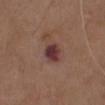The lesion was photographed on a routine skin check and not biopsied; there is no pathology result. This image is a 15 mm lesion crop taken from a total-body photograph. The tile uses white-light illumination. About 3 mm across. A male subject, about 75 years old. The total-body-photography lesion software estimated a lesion area of about 6 mm² and an eccentricity of roughly 0.6. And it measured border irregularity of about 1.5 on a 0–10 scale and a color-variation rating of about 4/10. The software also gave a classifier nevus-likeness of about 10/100 and a lesion-detection confidence of about 100/100. The lesion is on the chest.